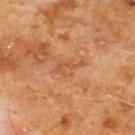| feature | finding |
|---|---|
| biopsy status | total-body-photography surveillance lesion; no biopsy |
| subject | male, aged 58–62 |
| lesion diameter | about 3.5 mm |
| location | the chest |
| imaging modality | total-body-photography crop, ~15 mm field of view |
| tile lighting | cross-polarized |